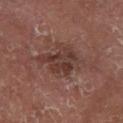Part of a total-body skin-imaging series; this lesion was reviewed on a skin check and was not flagged for biopsy. About 5.5 mm across. The patient is a male in their mid-60s. Imaged with cross-polarized lighting. The lesion is located on the leg. A 15 mm close-up tile from a total-body photography series done for melanoma screening.